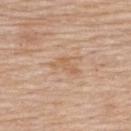{"biopsy_status": "not biopsied; imaged during a skin examination"}